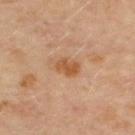{
  "biopsy_status": "not biopsied; imaged during a skin examination",
  "patient": {
    "sex": "female",
    "age_approx": 50
  },
  "site": "chest",
  "automated_metrics": {
    "area_mm2_approx": 4.5,
    "shape_asymmetry": 0.2,
    "cielab_L": 52,
    "cielab_a": 22,
    "cielab_b": 37,
    "vs_skin_darker_L": 9.0,
    "vs_skin_contrast_norm": 8.0,
    "border_irregularity_0_10": 2.0,
    "color_variation_0_10": 2.0,
    "peripheral_color_asymmetry": 0.5,
    "nevus_likeness_0_100": 40,
    "lesion_detection_confidence_0_100": 100
  },
  "image": {
    "source": "total-body photography crop",
    "field_of_view_mm": 15
  },
  "lesion_size": {
    "long_diameter_mm_approx": 3.0
  },
  "lighting": "cross-polarized"
}